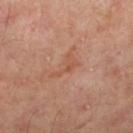The lesion was tiled from a total-body skin photograph and was not biopsied. The patient is a male aged around 50. On the leg. A 15 mm crop from a total-body photograph taken for skin-cancer surveillance.